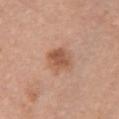Located on the chest. A close-up tile cropped from a whole-body skin photograph, about 15 mm across. This is a white-light tile. A female subject in their 60s.